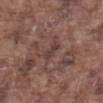Part of a total-body skin-imaging series; this lesion was reviewed on a skin check and was not flagged for biopsy.
From the abdomen.
This is a white-light tile.
A male patient aged approximately 75.
Automated tile analysis of the lesion measured a footprint of about 2.5 mm², an outline eccentricity of about 0.9 (0 = round, 1 = elongated), and a shape-asymmetry score of about 0.3 (0 = symmetric). The analysis additionally found an average lesion color of about L≈38 a*≈18 b*≈19 (CIELAB), a lesion–skin lightness drop of about 7, and a lesion-to-skin contrast of about 6.5 (normalized; higher = more distinct).
Measured at roughly 2.5 mm in maximum diameter.
Cropped from a total-body skin-imaging series; the visible field is about 15 mm.